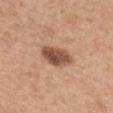biopsy status — catalogued during a skin exam; not biopsied | anatomic site — the mid back | image-analysis metrics — a lesion color around L≈51 a*≈22 b*≈29 in CIELAB, a lesion–skin lightness drop of about 15, and a lesion-to-skin contrast of about 10 (normalized; higher = more distinct); a border-irregularity rating of about 2.5/10 and a peripheral color-asymmetry measure near 2 | patient — male, in their 30s | imaging modality — total-body-photography crop, ~15 mm field of view.